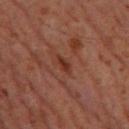workup: imaged on a skin check; not biopsied | subject: male, aged approximately 60 | tile lighting: cross-polarized | acquisition: ~15 mm crop, total-body skin-cancer survey | anatomic site: the left lower leg.